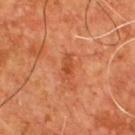This image is a 15 mm lesion crop taken from a total-body photograph. This is a cross-polarized tile. Automated tile analysis of the lesion measured a shape-asymmetry score of about 0.25 (0 = symmetric). The analysis additionally found a nevus-likeness score of about 0/100 and a lesion-detection confidence of about 100/100. The lesion is located on the chest. About 3 mm across. A male subject in their mid- to late 50s.